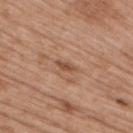Q: Was this lesion biopsied?
A: total-body-photography surveillance lesion; no biopsy
Q: What is the anatomic site?
A: the right upper arm
Q: What is the imaging modality?
A: 15 mm crop, total-body photography
Q: What lighting was used for the tile?
A: white-light
Q: Automated lesion metrics?
A: an outline eccentricity of about 0.9 (0 = round, 1 = elongated)
Q: What are the patient's age and sex?
A: female, aged approximately 40